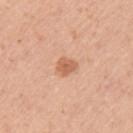No biopsy was performed on this lesion — it was imaged during a full skin examination and was not determined to be concerning. On the left upper arm. Measured at roughly 2.5 mm in maximum diameter. Imaged with white-light lighting. A 15 mm close-up extracted from a 3D total-body photography capture. The lesion-visualizer software estimated a mean CIELAB color near L≈61 a*≈25 b*≈35, a lesion–skin lightness drop of about 11, and a lesion-to-skin contrast of about 7 (normalized; higher = more distinct). The software also gave an automated nevus-likeness rating near 90 out of 100. A female patient about 30 years old.